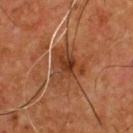| key | value |
|---|---|
| follow-up | no biopsy performed (imaged during a skin exam) |
| automated metrics | a footprint of about 12 mm², an outline eccentricity of about 0.45 (0 = round, 1 = elongated), and two-axis asymmetry of about 0.45; border irregularity of about 5 on a 0–10 scale and peripheral color asymmetry of about 1.5 |
| location | the chest |
| size | ~4.5 mm (longest diameter) |
| subject | male, aged around 55 |
| image source | ~15 mm tile from a whole-body skin photo |